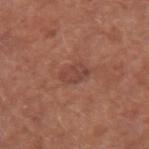follow-up: no biopsy performed (imaged during a skin exam)
image: total-body-photography crop, ~15 mm field of view
patient: male, about 60 years old
body site: the right forearm
illumination: white-light illumination
automated metrics: a footprint of about 5.5 mm², a shape eccentricity near 0.8, and two-axis asymmetry of about 0.25; a border-irregularity rating of about 2.5/10 and peripheral color asymmetry of about 0.5; a classifier nevus-likeness of about 5/100 and a lesion-detection confidence of about 100/100
lesion diameter: ≈3.5 mm